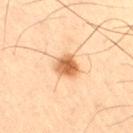The patient is a male about 65 years old. A lesion tile, about 15 mm wide, cut from a 3D total-body photograph. On the right thigh. An algorithmic analysis of the crop reported a footprint of about 6 mm² and a shape-asymmetry score of about 0.25 (0 = symmetric). It also reported a lesion-detection confidence of about 100/100. Captured under cross-polarized illumination. Longest diameter approximately 3 mm.